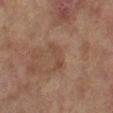No biopsy was performed on this lesion — it was imaged during a full skin examination and was not determined to be concerning.
The patient is a female roughly 60 years of age.
The recorded lesion diameter is about 3.5 mm.
The lesion is located on the left lower leg.
Cropped from a whole-body photographic skin survey; the tile spans about 15 mm.